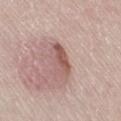* workup · imaged on a skin check; not biopsied
* location · the right thigh
* subject · male, roughly 75 years of age
* diameter · ≈4.5 mm
* image · total-body-photography crop, ~15 mm field of view